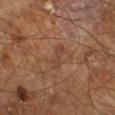notes = total-body-photography surveillance lesion; no biopsy | location = the leg | lesion size = ~3.5 mm (longest diameter) | patient = male, in their 60s | image-analysis metrics = a lesion area of about 4 mm², an eccentricity of roughly 0.9, and a symmetry-axis asymmetry near 0.25; a detector confidence of about 100 out of 100 that the crop contains a lesion | image = ~15 mm tile from a whole-body skin photo | lighting = cross-polarized.